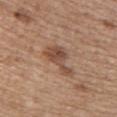Case summary:
– notes: total-body-photography surveillance lesion; no biopsy
– acquisition: ~15 mm crop, total-body skin-cancer survey
– anatomic site: the upper back
– automated lesion analysis: an average lesion color of about L≈49 a*≈19 b*≈29 (CIELAB) and about 10 CIELAB-L* units darker than the surrounding skin
– subject: female, roughly 60 years of age
– lesion size: about 5 mm
– illumination: white-light